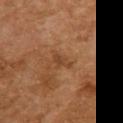Clinical impression: Imaged during a routine full-body skin examination; the lesion was not biopsied and no histopathology is available. Clinical summary: The patient is a female aged around 60. Imaged with cross-polarized lighting. This image is a 15 mm lesion crop taken from a total-body photograph. The recorded lesion diameter is about 2.5 mm. On the upper back.